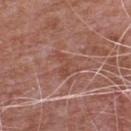Clinical impression: This lesion was catalogued during total-body skin photography and was not selected for biopsy.